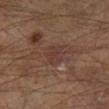notes — catalogued during a skin exam; not biopsied
subject — male, approximately 65 years of age
image — ~15 mm tile from a whole-body skin photo
body site — the right lower leg